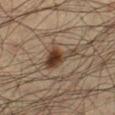The lesion was tiled from a total-body skin photograph and was not biopsied.
The subject is a male aged around 35.
A 15 mm crop from a total-body photograph taken for skin-cancer surveillance.
Automated tile analysis of the lesion measured a shape eccentricity near 0.85 and a symmetry-axis asymmetry near 0.55. And it measured a lesion color around L≈35 a*≈14 b*≈25 in CIELAB, about 12 CIELAB-L* units darker than the surrounding skin, and a normalized lesion–skin contrast near 11. The analysis additionally found radial color variation of about 1.5. And it measured a classifier nevus-likeness of about 95/100 and lesion-presence confidence of about 100/100.
Located on the leg.